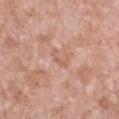{
  "biopsy_status": "not biopsied; imaged during a skin examination",
  "lighting": "white-light",
  "lesion_size": {
    "long_diameter_mm_approx": 2.5
  },
  "automated_metrics": {
    "border_irregularity_0_10": 6.0,
    "peripheral_color_asymmetry": 0.0,
    "nevus_likeness_0_100": 0
  },
  "patient": {
    "sex": "female",
    "age_approx": 75
  },
  "image": {
    "source": "total-body photography crop",
    "field_of_view_mm": 15
  },
  "site": "chest"
}